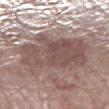This lesion was catalogued during total-body skin photography and was not selected for biopsy.
Longest diameter approximately 8.5 mm.
Captured under white-light illumination.
From the right lower leg.
A roughly 15 mm field-of-view crop from a total-body skin photograph.
The subject is a male aged approximately 70.
Automated tile analysis of the lesion measured an average lesion color of about L≈51 a*≈17 b*≈20 (CIELAB), a lesion–skin lightness drop of about 11, and a normalized border contrast of about 8. The software also gave a within-lesion color-variation index near 4.5/10 and radial color variation of about 1.5.